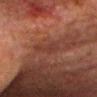notes=total-body-photography surveillance lesion; no biopsy
lesion size=~3 mm (longest diameter)
body site=the head or neck
subject=male, aged approximately 80
tile lighting=cross-polarized illumination
image source=total-body-photography crop, ~15 mm field of view